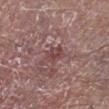  biopsy_status: not biopsied; imaged during a skin examination
  lighting: white-light
  site: left lower leg
  patient:
    sex: male
    age_approx: 65
  lesion_size:
    long_diameter_mm_approx: 2.5
  automated_metrics:
    cielab_L: 43
    cielab_a: 23
    cielab_b: 18
    vs_skin_darker_L: 8.0
    vs_skin_contrast_norm: 6.5
    border_irregularity_0_10: 2.0
    color_variation_0_10: 2.5
    nevus_likeness_0_100: 0
  image:
    source: total-body photography crop
    field_of_view_mm: 15